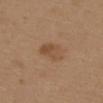Q: Was a biopsy performed?
A: imaged on a skin check; not biopsied
Q: Patient demographics?
A: female, approximately 40 years of age
Q: How was this image acquired?
A: ~15 mm tile from a whole-body skin photo
Q: What is the anatomic site?
A: the upper back
Q: Illumination type?
A: white-light
Q: Lesion size?
A: ~4 mm (longest diameter)